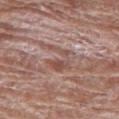workup: imaged on a skin check; not biopsied
acquisition: total-body-photography crop, ~15 mm field of view
body site: the left thigh
patient: female, aged 78–82